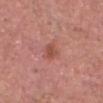Assessment: This lesion was catalogued during total-body skin photography and was not selected for biopsy. Image and clinical context: Imaged with white-light lighting. A male subject, in their 80s. The lesion's longest dimension is about 2.5 mm. Located on the head or neck. Cropped from a total-body skin-imaging series; the visible field is about 15 mm.